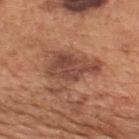imaging modality — total-body-photography crop, ~15 mm field of view
size — ~6.5 mm (longest diameter)
site — the upper back
tile lighting — white-light illumination
patient — male, aged 63 to 67